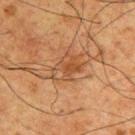Impression:
Captured during whole-body skin photography for melanoma surveillance; the lesion was not biopsied.
Context:
On the upper back. A male subject, approximately 60 years of age. A roughly 15 mm field-of-view crop from a total-body skin photograph.